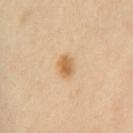Captured during whole-body skin photography for melanoma surveillance; the lesion was not biopsied. Measured at roughly 2.5 mm in maximum diameter. A female subject roughly 55 years of age. This image is a 15 mm lesion crop taken from a total-body photograph. Automated tile analysis of the lesion measured a border-irregularity index near 1.5/10, a within-lesion color-variation index near 4/10, and a peripheral color-asymmetry measure near 1.5. It also reported a nevus-likeness score of about 95/100 and a detector confidence of about 100 out of 100 that the crop contains a lesion. From the front of the torso.